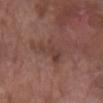biopsy_status: not biopsied; imaged during a skin examination
image:
  source: total-body photography crop
  field_of_view_mm: 15
automated_metrics:
  area_mm2_approx: 7.5
  eccentricity: 0.85
  shape_asymmetry: 0.45
  nevus_likeness_0_100: 0
  lesion_detection_confidence_0_100: 95
site: right forearm
lesion_size:
  long_diameter_mm_approx: 4.0
patient:
  sex: female
  age_approx: 70